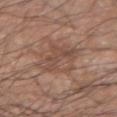| feature | finding |
|---|---|
| notes | imaged on a skin check; not biopsied |
| location | the left forearm |
| TBP lesion metrics | a shape eccentricity near 0.85 and a symmetry-axis asymmetry near 0.3; a border-irregularity index near 4/10, internal color variation of about 4.5 on a 0–10 scale, and peripheral color asymmetry of about 1.5; a nevus-likeness score of about 5/100 and a lesion-detection confidence of about 85/100 |
| patient | male, roughly 65 years of age |
| image source | 15 mm crop, total-body photography |
| lesion size | ≈6 mm |